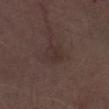{"patient": {"sex": "male", "age_approx": 70}, "image": {"source": "total-body photography crop", "field_of_view_mm": 15}, "site": "right thigh", "lesion_size": {"long_diameter_mm_approx": 3.0}}